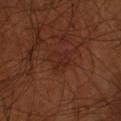Part of a total-body skin-imaging series; this lesion was reviewed on a skin check and was not flagged for biopsy.
A close-up tile cropped from a whole-body skin photograph, about 15 mm across.
The lesion is on the left forearm.
A male subject, aged 68 to 72.